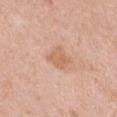workup = no biopsy performed (imaged during a skin exam); patient = female, aged approximately 40; anatomic site = the chest; image source = ~15 mm crop, total-body skin-cancer survey.